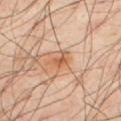{"biopsy_status": "not biopsied; imaged during a skin examination", "patient": {"sex": "male", "age_approx": 40}, "image": {"source": "total-body photography crop", "field_of_view_mm": 15}, "site": "back"}